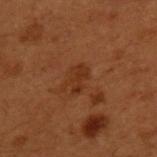biopsy status: total-body-photography surveillance lesion; no biopsy | lesion diameter: about 3 mm | automated lesion analysis: a nevus-likeness score of about 0/100 | subject: male, approximately 50 years of age | lighting: cross-polarized | site: the upper back | imaging modality: ~15 mm tile from a whole-body skin photo.